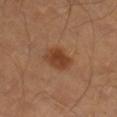A close-up tile cropped from a whole-body skin photograph, about 15 mm across.
A male patient aged around 40.
From the right lower leg.
Imaged with cross-polarized lighting.
An algorithmic analysis of the crop reported a border-irregularity index near 2/10, internal color variation of about 3.5 on a 0–10 scale, and a peripheral color-asymmetry measure near 1.
The recorded lesion diameter is about 3 mm.